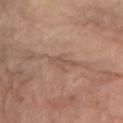Impression: The lesion was tiled from a total-body skin photograph and was not biopsied. Acquisition and patient details: Located on the left lower leg. This is a cross-polarized tile. The patient is a female aged approximately 65. A 15 mm close-up extracted from a 3D total-body photography capture. The total-body-photography lesion software estimated a footprint of about 3 mm², an outline eccentricity of about 0.9 (0 = round, 1 = elongated), and a shape-asymmetry score of about 0.3 (0 = symmetric). The software also gave a lesion color around L≈52 a*≈19 b*≈26 in CIELAB and a lesion-to-skin contrast of about 5 (normalized; higher = more distinct). The software also gave an automated nevus-likeness rating near 0 out of 100.